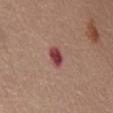location: the abdomen; patient: female, about 65 years old; illumination: white-light; acquisition: ~15 mm crop, total-body skin-cancer survey.